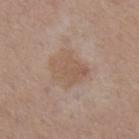Imaged during a routine full-body skin examination; the lesion was not biopsied and no histopathology is available. An algorithmic analysis of the crop reported a border-irregularity index near 3/10 and peripheral color asymmetry of about 1. A close-up tile cropped from a whole-body skin photograph, about 15 mm across. Approximately 4 mm at its widest. This is a white-light tile. From the chest. A male patient aged approximately 55.